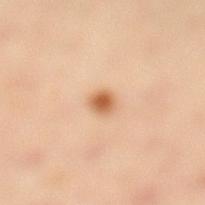This is a cross-polarized tile.
The subject is a female aged 38–42.
Cropped from a total-body skin-imaging series; the visible field is about 15 mm.
On the left lower leg.